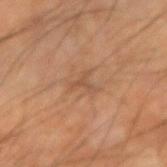Impression:
Recorded during total-body skin imaging; not selected for excision or biopsy.
Clinical summary:
Longest diameter approximately 3 mm. A male subject, aged around 60. Located on the arm. An algorithmic analysis of the crop reported about 6 CIELAB-L* units darker than the surrounding skin and a lesion-to-skin contrast of about 4.5 (normalized; higher = more distinct). Captured under cross-polarized illumination. A 15 mm close-up tile from a total-body photography series done for melanoma screening.